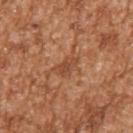biopsy_status: not biopsied; imaged during a skin examination
patient:
  sex: male
  age_approx: 45
image:
  source: total-body photography crop
  field_of_view_mm: 15
site: right upper arm
lighting: white-light
automated_metrics:
  vs_skin_darker_L: 8.0
  vs_skin_contrast_norm: 6.0
  nevus_likeness_0_100: 0
  lesion_detection_confidence_0_100: 80